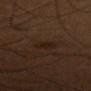About 2.5 mm across. A 15 mm crop from a total-body photograph taken for skin-cancer surveillance. Imaged with cross-polarized lighting. From the right thigh. The subject is a male aged 48 to 52.